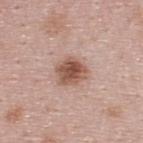Part of a total-body skin-imaging series; this lesion was reviewed on a skin check and was not flagged for biopsy.
Measured at roughly 3.5 mm in maximum diameter.
The subject is a male aged 23 to 27.
This image is a 15 mm lesion crop taken from a total-body photograph.
Captured under white-light illumination.
Located on the back.
The lesion-visualizer software estimated a lesion area of about 8.5 mm², a shape eccentricity near 0.5, and a shape-asymmetry score of about 0.25 (0 = symmetric). And it measured a lesion color around L≈53 a*≈21 b*≈27 in CIELAB, about 14 CIELAB-L* units darker than the surrounding skin, and a normalized border contrast of about 9.5. And it measured a lesion-detection confidence of about 100/100.